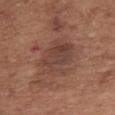| key | value |
|---|---|
| follow-up | total-body-photography surveillance lesion; no biopsy |
| patient | female, aged 73–77 |
| image | 15 mm crop, total-body photography |
| illumination | white-light |
| location | the chest |
| size | about 5 mm |
| automated metrics | an eccentricity of roughly 0.55; a classifier nevus-likeness of about 10/100 and a detector confidence of about 100 out of 100 that the crop contains a lesion |